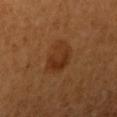This lesion was catalogued during total-body skin photography and was not selected for biopsy. The recorded lesion diameter is about 4 mm. On the arm. Captured under cross-polarized illumination. A 15 mm crop from a total-body photograph taken for skin-cancer surveillance. Automated tile analysis of the lesion measured a lesion color around L≈34 a*≈22 b*≈34 in CIELAB and a lesion-to-skin contrast of about 6.5 (normalized; higher = more distinct). The software also gave border irregularity of about 2.5 on a 0–10 scale and a color-variation rating of about 5/10. And it measured a nevus-likeness score of about 85/100 and lesion-presence confidence of about 100/100. The subject is a female aged approximately 55.